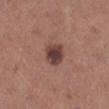  biopsy_status: not biopsied; imaged during a skin examination
  patient:
    sex: female
    age_approx: 65
  image:
    source: total-body photography crop
    field_of_view_mm: 15
  lighting: white-light
  automated_metrics:
    shape_asymmetry: 0.2
    border_irregularity_0_10: 2.0
    color_variation_0_10: 4.0
    peripheral_color_asymmetry: 1.0
    nevus_likeness_0_100: 55
    lesion_detection_confidence_0_100: 100
  lesion_size:
    long_diameter_mm_approx: 3.5
  site: right lower leg